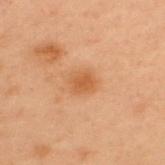Imaged during a routine full-body skin examination; the lesion was not biopsied and no histopathology is available. Imaged with cross-polarized lighting. Cropped from a whole-body photographic skin survey; the tile spans about 15 mm. From the back. The patient is a male in their mid-50s.